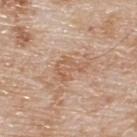No biopsy was performed on this lesion — it was imaged during a full skin examination and was not determined to be concerning. A lesion tile, about 15 mm wide, cut from a 3D total-body photograph. A male patient, aged 78 to 82. The lesion is on the upper back. Measured at roughly 3.5 mm in maximum diameter. The tile uses white-light illumination.